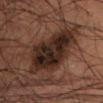No biopsy was performed on this lesion — it was imaged during a full skin examination and was not determined to be concerning. Captured under cross-polarized illumination. The lesion is located on the abdomen. The recorded lesion diameter is about 9 mm. The patient is a male about 60 years old. This image is a 15 mm lesion crop taken from a total-body photograph. The lesion-visualizer software estimated a footprint of about 28 mm², an outline eccentricity of about 0.85 (0 = round, 1 = elongated), and a shape-asymmetry score of about 0.3 (0 = symmetric). It also reported a lesion–skin lightness drop of about 12. And it measured a border-irregularity rating of about 4/10, internal color variation of about 6 on a 0–10 scale, and a peripheral color-asymmetry measure near 2. And it measured lesion-presence confidence of about 95/100.